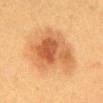Notes:
- biopsy status: no biopsy performed (imaged during a skin exam)
- imaging modality: ~15 mm crop, total-body skin-cancer survey
- lesion diameter: ≈7.5 mm
- site: the abdomen
- image-analysis metrics: an average lesion color of about L≈50 a*≈21 b*≈35 (CIELAB); an automated nevus-likeness rating near 90 out of 100
- lighting: cross-polarized illumination
- patient: female, roughly 40 years of age